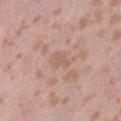Assessment: Part of a total-body skin-imaging series; this lesion was reviewed on a skin check and was not flagged for biopsy. Context: A 15 mm close-up extracted from a 3D total-body photography capture. On the right thigh. A female patient approximately 40 years of age.